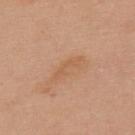| field | value |
|---|---|
| workup | imaged on a skin check; not biopsied |
| location | the upper back |
| TBP lesion metrics | a lesion–skin lightness drop of about 6 and a normalized border contrast of about 4.5; an automated nevus-likeness rating near 0 out of 100 |
| patient | female, in their mid-60s |
| image | ~15 mm tile from a whole-body skin photo |